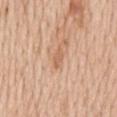| field | value |
|---|---|
| patient | male, aged 58 to 62 |
| anatomic site | the mid back |
| imaging modality | ~15 mm crop, total-body skin-cancer survey |
| lesion size | ≈3 mm |
| tile lighting | white-light illumination |